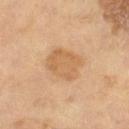Imaged during a routine full-body skin examination; the lesion was not biopsied and no histopathology is available. A male patient about 65 years old. The lesion is located on the leg. Automated tile analysis of the lesion measured an area of roughly 13 mm², an outline eccentricity of about 0.55 (0 = round, 1 = elongated), and two-axis asymmetry of about 0.15. It also reported a mean CIELAB color near L≈59 a*≈19 b*≈37 and a normalized lesion–skin contrast near 6. It also reported a border-irregularity rating of about 1.5/10, a within-lesion color-variation index near 2.5/10, and a peripheral color-asymmetry measure near 0.5. The analysis additionally found an automated nevus-likeness rating near 10 out of 100 and a detector confidence of about 100 out of 100 that the crop contains a lesion. Captured under cross-polarized illumination. Cropped from a total-body skin-imaging series; the visible field is about 15 mm.